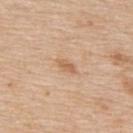Notes:
• follow-up — imaged on a skin check; not biopsied
• subject — male, aged 58 to 62
• body site — the back
• tile lighting — white-light illumination
• image source — ~15 mm crop, total-body skin-cancer survey